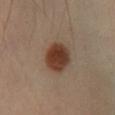Part of a total-body skin-imaging series; this lesion was reviewed on a skin check and was not flagged for biopsy.
Automated image analysis of the tile measured a lesion area of about 11 mm² and an outline eccentricity of about 0.45 (0 = round, 1 = elongated). The software also gave an automated nevus-likeness rating near 100 out of 100 and a lesion-detection confidence of about 100/100.
Cropped from a whole-body photographic skin survey; the tile spans about 15 mm.
On the right lower leg.
This is a cross-polarized tile.
Longest diameter approximately 4 mm.
The patient is a male aged around 40.